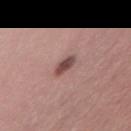{"biopsy_status": "not biopsied; imaged during a skin examination", "site": "abdomen", "image": {"source": "total-body photography crop", "field_of_view_mm": 15}, "lighting": "white-light", "patient": {"sex": "female", "age_approx": 25}, "lesion_size": {"long_diameter_mm_approx": 3.0}}